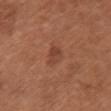Imaged during a routine full-body skin examination; the lesion was not biopsied and no histopathology is available.
A roughly 15 mm field-of-view crop from a total-body skin photograph.
On the chest.
The subject is a female in their mid- to late 60s.